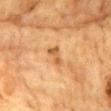Context:
Approximately 3 mm at its widest. From the front of the torso. A 15 mm close-up extracted from a 3D total-body photography capture. A male patient in their mid-80s or older. Automated tile analysis of the lesion measured an eccentricity of roughly 0.9 and two-axis asymmetry of about 0.4. The software also gave a border-irregularity index near 4.5/10, a within-lesion color-variation index near 0/10, and a peripheral color-asymmetry measure near 0. It also reported a detector confidence of about 100 out of 100 that the crop contains a lesion.